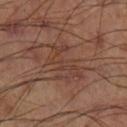workup: no biopsy performed (imaged during a skin exam) | lighting: cross-polarized illumination | lesion size: ≈5.5 mm | body site: the leg | TBP lesion metrics: an area of roughly 10 mm², an eccentricity of roughly 0.85, and a symmetry-axis asymmetry near 0.45; a mean CIELAB color near L≈40 a*≈21 b*≈26, about 6 CIELAB-L* units darker than the surrounding skin, and a normalized border contrast of about 5; lesion-presence confidence of about 65/100 | acquisition: ~15 mm tile from a whole-body skin photo.